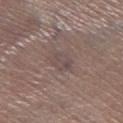{"biopsy_status": "not biopsied; imaged during a skin examination", "site": "right lower leg", "lighting": "white-light", "patient": {"sex": "female", "age_approx": 65}, "image": {"source": "total-body photography crop", "field_of_view_mm": 15}, "lesion_size": {"long_diameter_mm_approx": 3.0}}